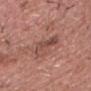Recorded during total-body skin imaging; not selected for excision or biopsy.
The lesion-visualizer software estimated a lesion color around L≈48 a*≈23 b*≈25 in CIELAB and a lesion–skin lightness drop of about 9. The software also gave a border-irregularity rating of about 6/10, a color-variation rating of about 7/10, and a peripheral color-asymmetry measure near 2.5.
Imaged with white-light lighting.
The patient is a male in their mid- to late 60s.
About 5 mm across.
A lesion tile, about 15 mm wide, cut from a 3D total-body photograph.
The lesion is located on the chest.